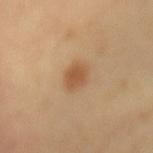Imaged during a routine full-body skin examination; the lesion was not biopsied and no histopathology is available.
A male subject in their mid- to late 60s.
Measured at roughly 2.5 mm in maximum diameter.
This image is a 15 mm lesion crop taken from a total-body photograph.
On the lower back.